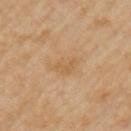Clinical impression: Imaged during a routine full-body skin examination; the lesion was not biopsied and no histopathology is available. Acquisition and patient details: The tile uses cross-polarized illumination. A 15 mm crop from a total-body photograph taken for skin-cancer surveillance. The patient is a female approximately 70 years of age. About 4 mm across. The lesion-visualizer software estimated border irregularity of about 3.5 on a 0–10 scale, a color-variation rating of about 2/10, and peripheral color asymmetry of about 0.5. On the left upper arm.